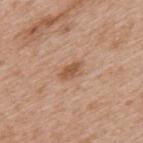The lesion was tiled from a total-body skin photograph and was not biopsied. A male subject, approximately 65 years of age. A 15 mm close-up tile from a total-body photography series done for melanoma screening. This is a white-light tile. Measured at roughly 3 mm in maximum diameter. From the upper back.